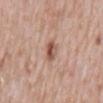On the mid back. A male patient approximately 50 years of age. Cropped from a total-body skin-imaging series; the visible field is about 15 mm.